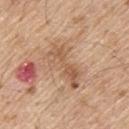{
  "site": "back",
  "image": {
    "source": "total-body photography crop",
    "field_of_view_mm": 15
  },
  "lighting": "white-light",
  "patient": {
    "sex": "male",
    "age_approx": 70
  }
}